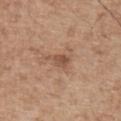Clinical impression:
This lesion was catalogued during total-body skin photography and was not selected for biopsy.
Context:
Longest diameter approximately 3 mm. The total-body-photography lesion software estimated an outline eccentricity of about 0.75 (0 = round, 1 = elongated). The software also gave peripheral color asymmetry of about 1. It also reported an automated nevus-likeness rating near 5 out of 100 and a detector confidence of about 100 out of 100 that the crop contains a lesion. A roughly 15 mm field-of-view crop from a total-body skin photograph. The tile uses white-light illumination. The lesion is on the right upper arm. The patient is a male roughly 70 years of age.